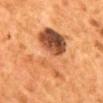biopsy status: total-body-photography surveillance lesion; no biopsy
patient: female, aged approximately 50
body site: the mid back
automated metrics: an outline eccentricity of about 0.7 (0 = round, 1 = elongated) and a shape-asymmetry score of about 0.5 (0 = symmetric); a normalized lesion–skin contrast near 8.5
lighting: cross-polarized
acquisition: ~15 mm tile from a whole-body skin photo
lesion size: ≈8 mm